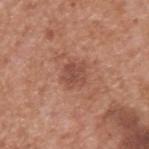{
  "automated_metrics": {
    "cielab_L": 49,
    "cielab_a": 24,
    "cielab_b": 28,
    "vs_skin_darker_L": 8.0,
    "vs_skin_contrast_norm": 6.0,
    "border_irregularity_0_10": 2.5,
    "color_variation_0_10": 3.0,
    "peripheral_color_asymmetry": 1.0
  },
  "site": "back",
  "lesion_size": {
    "long_diameter_mm_approx": 3.0
  },
  "patient": {
    "sex": "male",
    "age_approx": 65
  },
  "image": {
    "source": "total-body photography crop",
    "field_of_view_mm": 15
  },
  "lighting": "white-light"
}